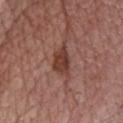biopsy_status: not biopsied; imaged during a skin examination
automated_metrics:
  border_irregularity_0_10: 3.0
  peripheral_color_asymmetry: 0.5
  nevus_likeness_0_100: 90
image:
  source: total-body photography crop
  field_of_view_mm: 15
patient:
  sex: male
  age_approx: 55
lesion_size:
  long_diameter_mm_approx: 4.0
lighting: white-light